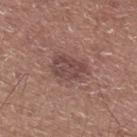<case>
  <biopsy_status>not biopsied; imaged during a skin examination</biopsy_status>
  <site>upper back</site>
  <patient>
    <sex>male</sex>
    <age_approx>60</age_approx>
  </patient>
  <image>
    <source>total-body photography crop</source>
    <field_of_view_mm>15</field_of_view_mm>
  </image>
</case>